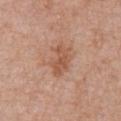Background:
About 4 mm across. The total-body-photography lesion software estimated a footprint of about 7 mm², an outline eccentricity of about 0.85 (0 = round, 1 = elongated), and a shape-asymmetry score of about 0.4 (0 = symmetric). And it measured an average lesion color of about L≈55 a*≈22 b*≈31 (CIELAB) and roughly 9 lightness units darker than nearby skin. This is a white-light tile. A region of skin cropped from a whole-body photographic capture, roughly 15 mm wide. From the abdomen. A male patient roughly 70 years of age.